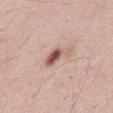Captured during whole-body skin photography for melanoma surveillance; the lesion was not biopsied. On the back. The total-body-photography lesion software estimated an area of roughly 5.5 mm², a shape eccentricity near 0.85, and a symmetry-axis asymmetry near 0.4. And it measured an average lesion color of about L≈57 a*≈20 b*≈26 (CIELAB) and a lesion-to-skin contrast of about 9 (normalized; higher = more distinct). The analysis additionally found a classifier nevus-likeness of about 85/100 and lesion-presence confidence of about 100/100. A close-up tile cropped from a whole-body skin photograph, about 15 mm across. The tile uses white-light illumination. Longest diameter approximately 3.5 mm. The patient is a male roughly 55 years of age.